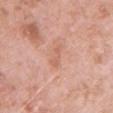The lesion was photographed on a routine skin check and not biopsied; there is no pathology result. Automated image analysis of the tile measured border irregularity of about 5 on a 0–10 scale and radial color variation of about 0. Longest diameter approximately 3.5 mm. Located on the chest. Captured under white-light illumination. A 15 mm crop from a total-body photograph taken for skin-cancer surveillance. The subject is a male aged 48 to 52.